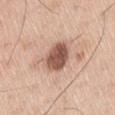Clinical impression: Imaged during a routine full-body skin examination; the lesion was not biopsied and no histopathology is available. Context: On the right thigh. Approximately 3.5 mm at its widest. This is a white-light tile. The patient is a male aged around 60. An algorithmic analysis of the crop reported a lesion area of about 10 mm² and an eccentricity of roughly 0.5. The software also gave a lesion color around L≈54 a*≈21 b*≈28 in CIELAB, a lesion–skin lightness drop of about 17, and a normalized border contrast of about 10.5. It also reported border irregularity of about 1.5 on a 0–10 scale, a color-variation rating of about 4/10, and radial color variation of about 1.5. It also reported a detector confidence of about 100 out of 100 that the crop contains a lesion. Cropped from a whole-body photographic skin survey; the tile spans about 15 mm.